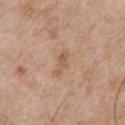Assessment:
Recorded during total-body skin imaging; not selected for excision or biopsy.
Clinical summary:
Cropped from a total-body skin-imaging series; the visible field is about 15 mm. The lesion is on the chest. A male subject, in their mid- to late 60s. About 3 mm across.